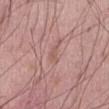This lesion was catalogued during total-body skin photography and was not selected for biopsy. On the abdomen. The lesion's longest dimension is about 2.5 mm. The tile uses white-light illumination. A close-up tile cropped from a whole-body skin photograph, about 15 mm across. A male patient, aged 53–57.